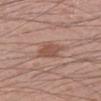Case summary:
– follow-up · no biopsy performed (imaged during a skin exam)
– lighting · white-light
– size · ~3 mm (longest diameter)
– patient · male, aged 53 to 57
– imaging modality · ~15 mm crop, total-body skin-cancer survey
– image-analysis metrics · border irregularity of about 2.5 on a 0–10 scale and a color-variation rating of about 3/10; a classifier nevus-likeness of about 15/100
– body site · the leg